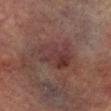| field | value |
|---|---|
| notes | no biopsy performed (imaged during a skin exam) |
| image source | ~15 mm tile from a whole-body skin photo |
| patient | male, aged 73–77 |
| location | the left lower leg |